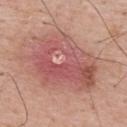biopsy_status: not biopsied; imaged during a skin examination
patient:
  sex: male
  age_approx: 65
site: back
automated_metrics:
  area_mm2_approx: 42.0
  eccentricity: 0.7
  lesion_detection_confidence_0_100: 95
lesion_size:
  long_diameter_mm_approx: 8.5
image:
  source: total-body photography crop
  field_of_view_mm: 15